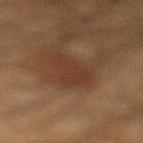<lesion>
  <biopsy_status>not biopsied; imaged during a skin examination</biopsy_status>
  <automated_metrics>
    <vs_skin_darker_L>5.0</vs_skin_darker_L>
    <vs_skin_contrast_norm>6.0</vs_skin_contrast_norm>
    <nevus_likeness_0_100>10</nevus_likeness_0_100>
    <lesion_detection_confidence_0_100>85</lesion_detection_confidence_0_100>
  </automated_metrics>
  <site>left lower leg</site>
  <patient>
    <sex>male</sex>
    <age_approx>35</age_approx>
  </patient>
  <lighting>cross-polarized</lighting>
  <lesion_size>
    <long_diameter_mm_approx>4.5</long_diameter_mm_approx>
  </lesion_size>
  <image>
    <source>total-body photography crop</source>
    <field_of_view_mm>15</field_of_view_mm>
  </image>
</lesion>